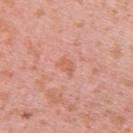Recorded during total-body skin imaging; not selected for excision or biopsy.
About 2.5 mm across.
The lesion is located on the upper back.
The patient is a female aged around 40.
Automated image analysis of the tile measured a footprint of about 3.5 mm², a shape eccentricity near 0.75, and two-axis asymmetry of about 0.4. And it measured a border-irregularity rating of about 3.5/10, internal color variation of about 1.5 on a 0–10 scale, and a peripheral color-asymmetry measure near 0.5. It also reported a nevus-likeness score of about 0/100 and lesion-presence confidence of about 100/100.
A region of skin cropped from a whole-body photographic capture, roughly 15 mm wide.